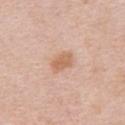Q: Is there a histopathology result?
A: catalogued during a skin exam; not biopsied
Q: What are the patient's age and sex?
A: female, roughly 45 years of age
Q: What is the lesion's diameter?
A: ≈3 mm
Q: What is the imaging modality?
A: total-body-photography crop, ~15 mm field of view
Q: Illumination type?
A: white-light
Q: What is the anatomic site?
A: the upper back